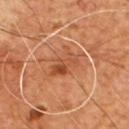– notes · catalogued during a skin exam; not biopsied
– location · the chest
– imaging modality · ~15 mm tile from a whole-body skin photo
– diameter · ≈4 mm
– subject · male, aged 53–57
– automated metrics · a color-variation rating of about 7/10 and radial color variation of about 3